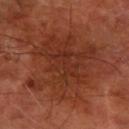Case summary:
- biopsy status — imaged on a skin check; not biopsied
- patient — aged around 65
- size — about 8 mm
- tile lighting — cross-polarized
- image source — total-body-photography crop, ~15 mm field of view
- anatomic site — the right forearm